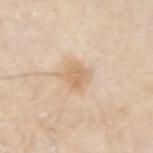workup = no biopsy performed (imaged during a skin exam); subject = male, approximately 80 years of age; location = the left forearm; lesion size = about 3 mm; lighting = white-light; imaging modality = ~15 mm crop, total-body skin-cancer survey.